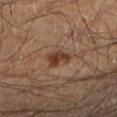Findings:
– biopsy status — total-body-photography surveillance lesion; no biopsy
– diameter — ~3 mm (longest diameter)
– automated metrics — a footprint of about 6.5 mm²; an average lesion color of about L≈35 a*≈18 b*≈26 (CIELAB) and a lesion–skin lightness drop of about 9; a detector confidence of about 100 out of 100 that the crop contains a lesion
– location — the left lower leg
– acquisition — ~15 mm tile from a whole-body skin photo
– patient — male, in their 60s
– illumination — cross-polarized illumination